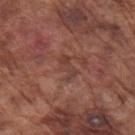Assessment:
Captured during whole-body skin photography for melanoma surveillance; the lesion was not biopsied.
Clinical summary:
A roughly 15 mm field-of-view crop from a total-body skin photograph. A male patient, in their mid-70s. This is a white-light tile. The total-body-photography lesion software estimated a lesion–skin lightness drop of about 6 and a normalized border contrast of about 5.5. And it measured an automated nevus-likeness rating near 0 out of 100 and a lesion-detection confidence of about 80/100. The lesion is located on the right upper arm. About 3.5 mm across.